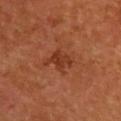Assessment:
The lesion was tiled from a total-body skin photograph and was not biopsied.
Clinical summary:
The lesion is located on the back. A male patient aged around 55. This is a cross-polarized tile. A close-up tile cropped from a whole-body skin photograph, about 15 mm across.